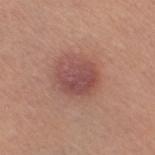Acquisition and patient details:
Measured at roughly 4.5 mm in maximum diameter. An algorithmic analysis of the crop reported an eccentricity of roughly 0.5 and a symmetry-axis asymmetry near 0.15. And it measured border irregularity of about 2 on a 0–10 scale and a peripheral color-asymmetry measure near 1.5. The software also gave a nevus-likeness score of about 95/100 and a detector confidence of about 100 out of 100 that the crop contains a lesion. The lesion is located on the right thigh. The subject is a female aged 43 to 47. A 15 mm close-up tile from a total-body photography series done for melanoma screening.